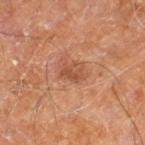Assessment: No biopsy was performed on this lesion — it was imaged during a full skin examination and was not determined to be concerning. Context: The lesion's longest dimension is about 2.5 mm. Cropped from a total-body skin-imaging series; the visible field is about 15 mm. The tile uses cross-polarized illumination. An algorithmic analysis of the crop reported a lesion color around L≈49 a*≈24 b*≈33 in CIELAB and about 8 CIELAB-L* units darker than the surrounding skin. Located on the right leg. The patient is a male aged 58 to 62.